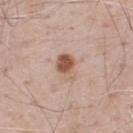Clinical impression: The lesion was tiled from a total-body skin photograph and was not biopsied. Clinical summary: Longest diameter approximately 3 mm. A 15 mm close-up tile from a total-body photography series done for melanoma screening. From the upper back. Captured under white-light illumination. The total-body-photography lesion software estimated a footprint of about 6.5 mm², an outline eccentricity of about 0.7 (0 = round, 1 = elongated), and a symmetry-axis asymmetry near 0.25. The software also gave a lesion color around L≈57 a*≈19 b*≈28 in CIELAB, roughly 12 lightness units darker than nearby skin, and a lesion-to-skin contrast of about 8.5 (normalized; higher = more distinct). The analysis additionally found a border-irregularity index near 2/10, a within-lesion color-variation index near 9/10, and a peripheral color-asymmetry measure near 3.5. The software also gave lesion-presence confidence of about 100/100. A male patient about 70 years old.